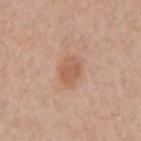| feature | finding |
|---|---|
| follow-up | total-body-photography surveillance lesion; no biopsy |
| imaging modality | 15 mm crop, total-body photography |
| diameter | ~3 mm (longest diameter) |
| anatomic site | the right upper arm |
| illumination | white-light |
| patient | male, aged 58 to 62 |